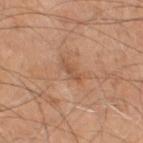Impression:
No biopsy was performed on this lesion — it was imaged during a full skin examination and was not determined to be concerning.
Acquisition and patient details:
About 3 mm across. The lesion-visualizer software estimated a mean CIELAB color near L≈51 a*≈21 b*≈32 and a lesion–skin lightness drop of about 8. The subject is a male aged around 70. This is a cross-polarized tile. This image is a 15 mm lesion crop taken from a total-body photograph. The lesion is located on the left thigh.